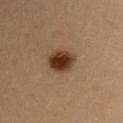A lesion tile, about 15 mm wide, cut from a 3D total-body photograph.
On the back.
Captured under cross-polarized illumination.
The recorded lesion diameter is about 3.5 mm.
A female patient, roughly 35 years of age.
The lesion-visualizer software estimated a footprint of about 8 mm², an eccentricity of roughly 0.6, and two-axis asymmetry of about 0.15.